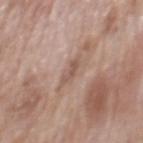Findings:
• subject · male, roughly 70 years of age
• illumination · white-light illumination
• acquisition · total-body-photography crop, ~15 mm field of view
• lesion size · about 2.5 mm
• body site · the mid back